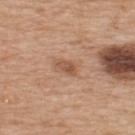notes — no biopsy performed (imaged during a skin exam) | acquisition — total-body-photography crop, ~15 mm field of view | illumination — white-light illumination | automated metrics — an automated nevus-likeness rating near 25 out of 100 and a lesion-detection confidence of about 100/100 | lesion size — ≈2.5 mm | patient — male, in their mid- to late 60s | site — the upper back.